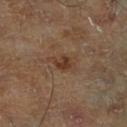| feature | finding |
|---|---|
| workup | imaged on a skin check; not biopsied |
| image source | ~15 mm tile from a whole-body skin photo |
| patient | male, aged 68–72 |
| body site | the right lower leg |